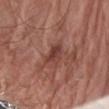A male subject aged 78 to 82.
A lesion tile, about 15 mm wide, cut from a 3D total-body photograph.
An algorithmic analysis of the crop reported a classifier nevus-likeness of about 0/100 and a detector confidence of about 95 out of 100 that the crop contains a lesion.
Imaged with white-light lighting.
The lesion is on the right forearm.
The recorded lesion diameter is about 3.5 mm.